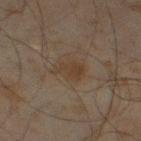Clinical impression:
No biopsy was performed on this lesion — it was imaged during a full skin examination and was not determined to be concerning.
Background:
Imaged with cross-polarized lighting. The patient is a male roughly 45 years of age. A 15 mm crop from a total-body photograph taken for skin-cancer surveillance. Located on the left thigh.